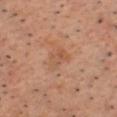Assessment:
No biopsy was performed on this lesion — it was imaged during a full skin examination and was not determined to be concerning.
Image and clinical context:
A male subject aged 53 to 57. The lesion is located on the chest. A 15 mm close-up tile from a total-body photography series done for melanoma screening.